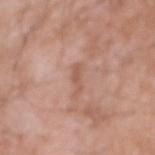Captured during whole-body skin photography for melanoma surveillance; the lesion was not biopsied. A male patient, in their 60s. A region of skin cropped from a whole-body photographic capture, roughly 15 mm wide. Captured under white-light illumination. The lesion is located on the right forearm.